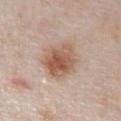site — the chest | tile lighting — white-light | image source — 15 mm crop, total-body photography | subject — male, aged around 75 | TBP lesion metrics — a lesion–skin lightness drop of about 12 and a normalized border contrast of about 8.5; a classifier nevus-likeness of about 95/100 and a lesion-detection confidence of about 100/100 | lesion diameter — about 5 mm.